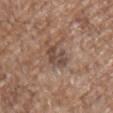No biopsy was performed on this lesion — it was imaged during a full skin examination and was not determined to be concerning.
A male patient, in their 70s.
The lesion is on the left upper arm.
Automated tile analysis of the lesion measured an area of roughly 6.5 mm², an outline eccentricity of about 0.55 (0 = round, 1 = elongated), and a shape-asymmetry score of about 0.25 (0 = symmetric).
A 15 mm close-up tile from a total-body photography series done for melanoma screening.
The tile uses white-light illumination.
The recorded lesion diameter is about 3.5 mm.